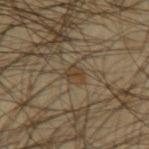  biopsy_status: not biopsied; imaged during a skin examination
  lesion_size:
    long_diameter_mm_approx: 2.5
  patient:
    sex: male
    age_approx: 45
  site: left upper arm
  image:
    source: total-body photography crop
    field_of_view_mm: 15
  lighting: cross-polarized